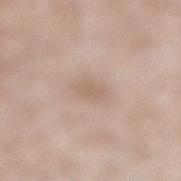Assessment: Recorded during total-body skin imaging; not selected for excision or biopsy.